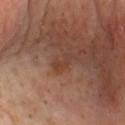Captured during whole-body skin photography for melanoma surveillance; the lesion was not biopsied. Longest diameter approximately 2.5 mm. This image is a 15 mm lesion crop taken from a total-body photograph. A male subject, in their mid- to late 60s. The lesion is on the chest. The total-body-photography lesion software estimated roughly 6 lightness units darker than nearby skin. The software also gave lesion-presence confidence of about 100/100. Imaged with cross-polarized lighting.